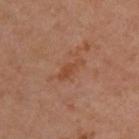Part of a total-body skin-imaging series; this lesion was reviewed on a skin check and was not flagged for biopsy. The total-body-photography lesion software estimated a lesion–skin lightness drop of about 6 and a normalized lesion–skin contrast near 6. The software also gave a border-irregularity index near 4.5/10 and radial color variation of about 0.5. It also reported a nevus-likeness score of about 0/100 and a lesion-detection confidence of about 100/100. From the back. A female subject about 45 years old. This is a cross-polarized tile. A 15 mm crop from a total-body photograph taken for skin-cancer surveillance.